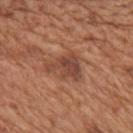Q: Was this lesion biopsied?
A: no biopsy performed (imaged during a skin exam)
Q: What is the anatomic site?
A: the mid back
Q: What is the imaging modality?
A: total-body-photography crop, ~15 mm field of view
Q: Patient demographics?
A: male, roughly 65 years of age